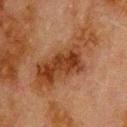Q: Is there a histopathology result?
A: imaged on a skin check; not biopsied
Q: What did automated image analysis measure?
A: a lesion color around L≈32 a*≈20 b*≈29 in CIELAB, about 9 CIELAB-L* units darker than the surrounding skin, and a normalized border contrast of about 9; a border-irregularity rating of about 7.5/10 and a peripheral color-asymmetry measure near 1.5; a classifier nevus-likeness of about 0/100 and a detector confidence of about 100 out of 100 that the crop contains a lesion
Q: Lesion location?
A: the upper back
Q: Who is the patient?
A: male, roughly 80 years of age
Q: What kind of image is this?
A: ~15 mm tile from a whole-body skin photo
Q: How was the tile lit?
A: cross-polarized